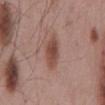Notes:
* follow-up — total-body-photography surveillance lesion; no biopsy
* diameter — about 4.5 mm
* image — ~15 mm crop, total-body skin-cancer survey
* image-analysis metrics — a lesion area of about 7.5 mm² and a shape eccentricity near 0.85; a lesion color around L≈47 a*≈21 b*≈25 in CIELAB, about 11 CIELAB-L* units darker than the surrounding skin, and a lesion-to-skin contrast of about 8 (normalized; higher = more distinct); border irregularity of about 2.5 on a 0–10 scale; a classifier nevus-likeness of about 90/100 and lesion-presence confidence of about 100/100
* location — the mid back
* subject — male, in their mid-50s